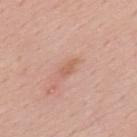Cropped from a total-body skin-imaging series; the visible field is about 15 mm. Longest diameter approximately 2.5 mm. A male patient, about 50 years old. On the back. Automated image analysis of the tile measured a lesion area of about 2.5 mm², an outline eccentricity of about 0.9 (0 = round, 1 = elongated), and two-axis asymmetry of about 0.3. It also reported border irregularity of about 3 on a 0–10 scale and internal color variation of about 0 on a 0–10 scale. It also reported a lesion-detection confidence of about 100/100. Imaged with white-light lighting.